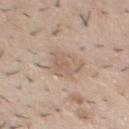follow-up: no biopsy performed (imaged during a skin exam) | subject: male, aged 28–32 | body site: the chest | automated metrics: a lesion color around L≈61 a*≈15 b*≈27 in CIELAB, about 7 CIELAB-L* units darker than the surrounding skin, and a normalized border contrast of about 5; a border-irregularity index near 3/10, a within-lesion color-variation index near 3/10, and a peripheral color-asymmetry measure near 1 | image source: ~15 mm crop, total-body skin-cancer survey.